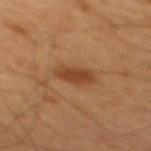  biopsy_status: not biopsied; imaged during a skin examination
  site: mid back
  lighting: cross-polarized
  patient:
    sex: male
    age_approx: 65
  lesion_size:
    long_diameter_mm_approx: 3.5
  automated_metrics:
    area_mm2_approx: 6.5
    eccentricity: 0.8
    shape_asymmetry: 0.15
    nevus_likeness_0_100: 95
    lesion_detection_confidence_0_100: 100
  image:
    source: total-body photography crop
    field_of_view_mm: 15